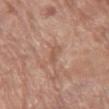Clinical impression:
The lesion was tiled from a total-body skin photograph and was not biopsied.
Acquisition and patient details:
The recorded lesion diameter is about 3 mm. A female patient aged 73–77. A lesion tile, about 15 mm wide, cut from a 3D total-body photograph. The lesion is located on the right thigh.